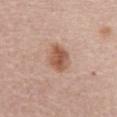Clinical impression: Recorded during total-body skin imaging; not selected for excision or biopsy. Acquisition and patient details: Cropped from a total-body skin-imaging series; the visible field is about 15 mm. Located on the abdomen. The patient is a female roughly 65 years of age.